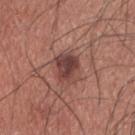| field | value |
|---|---|
| notes | imaged on a skin check; not biopsied |
| illumination | white-light |
| image-analysis metrics | an area of roughly 8 mm², an outline eccentricity of about 0.7 (0 = round, 1 = elongated), and two-axis asymmetry of about 0.2; a border-irregularity rating of about 2.5/10, a color-variation rating of about 4.5/10, and radial color variation of about 1.5; a nevus-likeness score of about 80/100 and a lesion-detection confidence of about 100/100 |
| imaging modality | 15 mm crop, total-body photography |
| patient | male, aged 33–37 |
| anatomic site | the upper back |